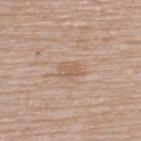<lesion>
  <biopsy_status>not biopsied; imaged during a skin examination</biopsy_status>
  <lighting>white-light</lighting>
  <patient>
    <sex>male</sex>
    <age_approx>65</age_approx>
  </patient>
  <image>
    <source>total-body photography crop</source>
    <field_of_view_mm>15</field_of_view_mm>
  </image>
  <site>upper back</site>
</lesion>